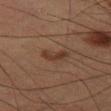Impression: Recorded during total-body skin imaging; not selected for excision or biopsy. Acquisition and patient details: Automated tile analysis of the lesion measured a footprint of about 4.5 mm², a shape eccentricity near 0.9, and a symmetry-axis asymmetry near 0.3. And it measured a lesion-detection confidence of about 100/100. A male patient aged 53 to 57. Measured at roughly 3 mm in maximum diameter. Cropped from a whole-body photographic skin survey; the tile spans about 15 mm. The lesion is on the right thigh. Imaged with cross-polarized lighting.